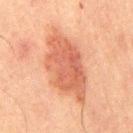The lesion was tiled from a total-body skin photograph and was not biopsied. A 15 mm close-up tile from a total-body photography series done for melanoma screening. The total-body-photography lesion software estimated a border-irregularity rating of about 3/10 and a color-variation rating of about 3.5/10. It also reported a detector confidence of about 100 out of 100 that the crop contains a lesion. Longest diameter approximately 8.5 mm. This is a cross-polarized tile. The lesion is located on the back. The subject is a male aged 63 to 67.